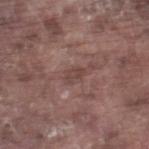Impression:
The lesion was tiled from a total-body skin photograph and was not biopsied.
Clinical summary:
On the right lower leg. A male subject about 75 years old. Imaged with white-light lighting. A close-up tile cropped from a whole-body skin photograph, about 15 mm across. The lesion-visualizer software estimated a lesion color around L≈43 a*≈19 b*≈21 in CIELAB and a lesion-to-skin contrast of about 6 (normalized; higher = more distinct). The analysis additionally found an automated nevus-likeness rating near 0 out of 100.